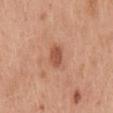Q: Was this lesion biopsied?
A: no biopsy performed (imaged during a skin exam)
Q: What is the imaging modality?
A: ~15 mm tile from a whole-body skin photo
Q: What is the lesion's diameter?
A: ~2.5 mm (longest diameter)
Q: What is the anatomic site?
A: the mid back
Q: Who is the patient?
A: male, aged approximately 30
Q: Illumination type?
A: white-light